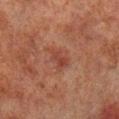Q: Is there a histopathology result?
A: total-body-photography surveillance lesion; no biopsy